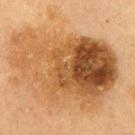follow-up — catalogued during a skin exam; not biopsied | illumination — cross-polarized illumination | subject — female, about 60 years old | image source — ~15 mm tile from a whole-body skin photo | lesion size — ~12.5 mm (longest diameter) | site — the mid back.